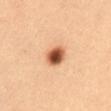The lesion was photographed on a routine skin check and not biopsied; there is no pathology result. This image is a 15 mm lesion crop taken from a total-body photograph. From the abdomen. The subject is a female about 35 years old. The recorded lesion diameter is about 3 mm. Captured under cross-polarized illumination.